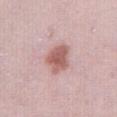notes = catalogued during a skin exam; not biopsied.